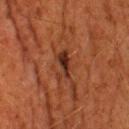biopsy status: catalogued during a skin exam; not biopsied
site: the back
lesion diameter: about 3.5 mm
illumination: cross-polarized
patient: male, aged 58–62
imaging modality: ~15 mm crop, total-body skin-cancer survey